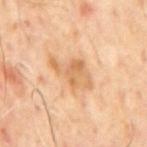biopsy status: total-body-photography surveillance lesion; no biopsy | subject: male, aged 63–67 | body site: the mid back | image-analysis metrics: a lesion area of about 9.5 mm² and an outline eccentricity of about 0.85 (0 = round, 1 = elongated); an average lesion color of about L≈65 a*≈20 b*≈39 (CIELAB) and a normalized lesion–skin contrast near 6.5; an automated nevus-likeness rating near 0 out of 100 and lesion-presence confidence of about 100/100 | lesion size: ~5.5 mm (longest diameter) | image: ~15 mm tile from a whole-body skin photo.